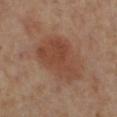Imaged during a routine full-body skin examination; the lesion was not biopsied and no histopathology is available. The lesion's longest dimension is about 6.5 mm. A lesion tile, about 15 mm wide, cut from a 3D total-body photograph. On the left lower leg. Imaged with cross-polarized lighting. The patient is a female in their mid-50s.